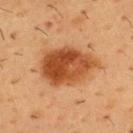Acquisition and patient details: About 7 mm across. A male patient in their mid-50s. Automated tile analysis of the lesion measured an average lesion color of about L≈43 a*≈24 b*≈36 (CIELAB), about 13 CIELAB-L* units darker than the surrounding skin, and a normalized border contrast of about 10.5. The analysis additionally found a border-irregularity rating of about 2/10 and internal color variation of about 6 on a 0–10 scale. The software also gave a classifier nevus-likeness of about 100/100. On the front of the torso. A close-up tile cropped from a whole-body skin photograph, about 15 mm across.